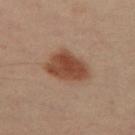Captured during whole-body skin photography for melanoma surveillance; the lesion was not biopsied. A female patient, in their 40s. A 15 mm close-up tile from a total-body photography series done for melanoma screening. The lesion's longest dimension is about 5 mm. The lesion is on the right leg.